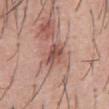follow-up: total-body-photography surveillance lesion; no biopsy
tile lighting: white-light
patient: male, in their mid-50s
site: the abdomen
acquisition: total-body-photography crop, ~15 mm field of view
automated metrics: an average lesion color of about L≈51 a*≈23 b*≈25 (CIELAB), about 11 CIELAB-L* units darker than the surrounding skin, and a lesion-to-skin contrast of about 7.5 (normalized; higher = more distinct)